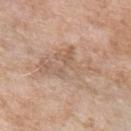{"biopsy_status": "not biopsied; imaged during a skin examination", "patient": {"sex": "female", "age_approx": 75}, "image": {"source": "total-body photography crop", "field_of_view_mm": 15}, "site": "left upper arm", "lesion_size": {"long_diameter_mm_approx": 8.0}, "lighting": "white-light", "automated_metrics": {"vs_skin_darker_L": 7.0, "vs_skin_contrast_norm": 5.0, "color_variation_0_10": 4.5, "peripheral_color_asymmetry": 1.5, "nevus_likeness_0_100": 0, "lesion_detection_confidence_0_100": 85}}